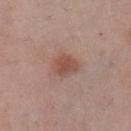| feature | finding |
|---|---|
| image-analysis metrics | an area of roughly 6.5 mm², an eccentricity of roughly 0.65, and a symmetry-axis asymmetry near 0.25; internal color variation of about 2.5 on a 0–10 scale and a peripheral color-asymmetry measure near 0.5; a nevus-likeness score of about 85/100 |
| patient | female, aged approximately 50 |
| illumination | white-light illumination |
| site | the left lower leg |
| image | 15 mm crop, total-body photography |
| lesion size | ≈3.5 mm |